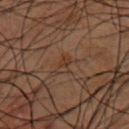<lesion>
<biopsy_status>not biopsied; imaged during a skin examination</biopsy_status>
<lesion_size>
  <long_diameter_mm_approx>2.5</long_diameter_mm_approx>
</lesion_size>
<lighting>cross-polarized</lighting>
<patient>
  <sex>male</sex>
  <age_approx>50</age_approx>
</patient>
<automated_metrics>
  <area_mm2_approx>3.0</area_mm2_approx>
  <eccentricity>0.85</eccentricity>
  <shape_asymmetry>0.35</shape_asymmetry>
  <cielab_L>26</cielab_L>
  <cielab_a>14</cielab_a>
  <cielab_b>22</cielab_b>
  <vs_skin_darker_L>4.0</vs_skin_darker_L>
  <vs_skin_contrast_norm>5.5</vs_skin_contrast_norm>
  <border_irregularity_0_10>3.0</border_irregularity_0_10>
  <color_variation_0_10>3.5</color_variation_0_10>
  <peripheral_color_asymmetry>1.0</peripheral_color_asymmetry>
  <nevus_likeness_0_100>0</nevus_likeness_0_100>
  <lesion_detection_confidence_0_100>100</lesion_detection_confidence_0_100>
</automated_metrics>
<image>
  <source>total-body photography crop</source>
  <field_of_view_mm>15</field_of_view_mm>
</image>
<site>chest</site>
</lesion>